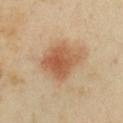Notes:
* workup — catalogued during a skin exam; not biopsied
* automated lesion analysis — a lesion color around L≈58 a*≈21 b*≈35 in CIELAB, about 11 CIELAB-L* units darker than the surrounding skin, and a lesion-to-skin contrast of about 7.5 (normalized; higher = more distinct)
* imaging modality — total-body-photography crop, ~15 mm field of view
* body site — the chest
* lesion diameter — about 5.5 mm
* patient — female, aged 38–42